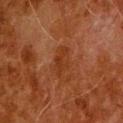Notes:
- biopsy status: total-body-photography surveillance lesion; no biopsy
- automated metrics: a mean CIELAB color near L≈28 a*≈22 b*≈30, a lesion–skin lightness drop of about 5, and a lesion-to-skin contrast of about 6 (normalized; higher = more distinct)
- tile lighting: cross-polarized illumination
- imaging modality: ~15 mm crop, total-body skin-cancer survey
- lesion size: about 3.5 mm
- site: the head or neck
- subject: male, aged 78 to 82